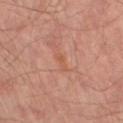follow-up — total-body-photography surveillance lesion; no biopsy
site — the left thigh
image source — ~15 mm crop, total-body skin-cancer survey
lesion size — ~2.5 mm (longest diameter)
patient — male, aged approximately 70